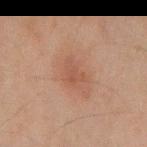This lesion was catalogued during total-body skin photography and was not selected for biopsy.
The lesion's longest dimension is about 3 mm.
Imaged with cross-polarized lighting.
The total-body-photography lesion software estimated a mean CIELAB color near L≈42 a*≈19 b*≈25, roughly 6 lightness units darker than nearby skin, and a normalized lesion–skin contrast near 5. The analysis additionally found border irregularity of about 5 on a 0–10 scale and a peripheral color-asymmetry measure near 0.5. And it measured a classifier nevus-likeness of about 15/100 and lesion-presence confidence of about 100/100.
A male subject, aged 43 to 47.
A close-up tile cropped from a whole-body skin photograph, about 15 mm across.
Located on the mid back.